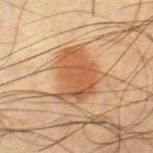Captured during whole-body skin photography for melanoma surveillance; the lesion was not biopsied. The recorded lesion diameter is about 6 mm. Cropped from a whole-body photographic skin survey; the tile spans about 15 mm. A male subject aged around 65. Located on the right thigh.